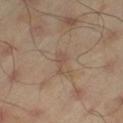biopsy status = catalogued during a skin exam; not biopsied
acquisition = ~15 mm crop, total-body skin-cancer survey
body site = the leg
patient = male, aged around 45